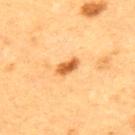biopsy status: total-body-photography surveillance lesion; no biopsy | patient: male, aged approximately 50 | body site: the upper back | tile lighting: cross-polarized illumination | automated lesion analysis: an outline eccentricity of about 0.85 (0 = round, 1 = elongated) and two-axis asymmetry of about 0.2; a lesion color around L≈51 a*≈24 b*≈41 in CIELAB, roughly 13 lightness units darker than nearby skin, and a normalized border contrast of about 9.5; border irregularity of about 2 on a 0–10 scale, internal color variation of about 3 on a 0–10 scale, and peripheral color asymmetry of about 1 | lesion size: ≈3 mm | acquisition: total-body-photography crop, ~15 mm field of view.